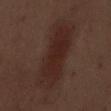| key | value |
|---|---|
| subject | male, in their 70s |
| body site | the abdomen |
| lighting | white-light illumination |
| image | total-body-photography crop, ~15 mm field of view |
| TBP lesion metrics | a footprint of about 28 mm², an outline eccentricity of about 0.9 (0 = round, 1 = elongated), and a shape-asymmetry score of about 0.15 (0 = symmetric) |